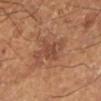Imaged during a routine full-body skin examination; the lesion was not biopsied and no histopathology is available.
A male patient, aged 63 to 67.
Cropped from a whole-body photographic skin survey; the tile spans about 15 mm.
The tile uses cross-polarized illumination.
The lesion is on the left lower leg.